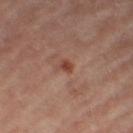Clinical impression: No biopsy was performed on this lesion — it was imaged during a full skin examination and was not determined to be concerning. Acquisition and patient details: The tile uses cross-polarized illumination. Measured at roughly 1.5 mm in maximum diameter. A 15 mm crop from a total-body photograph taken for skin-cancer surveillance. A female patient aged 58–62. Automated tile analysis of the lesion measured a shape-asymmetry score of about 0.2 (0 = symmetric). It also reported an average lesion color of about L≈44 a*≈24 b*≈29 (CIELAB), about 10 CIELAB-L* units darker than the surrounding skin, and a normalized border contrast of about 8. Located on the right thigh.